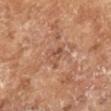Q: Was a biopsy performed?
A: catalogued during a skin exam; not biopsied
Q: What is the lesion's diameter?
A: about 3 mm
Q: Who is the patient?
A: male, in their mid- to late 60s
Q: What is the imaging modality?
A: ~15 mm tile from a whole-body skin photo
Q: How was the tile lit?
A: cross-polarized illumination
Q: Automated lesion metrics?
A: a mean CIELAB color near L≈51 a*≈21 b*≈30, about 7 CIELAB-L* units darker than the surrounding skin, and a lesion-to-skin contrast of about 5 (normalized; higher = more distinct); an automated nevus-likeness rating near 0 out of 100 and a detector confidence of about 100 out of 100 that the crop contains a lesion